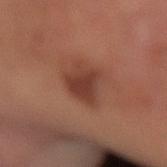follow-up: imaged on a skin check; not biopsied
lesion size: about 4 mm
automated lesion analysis: a footprint of about 9.5 mm², a shape eccentricity near 0.55, and two-axis asymmetry of about 0.25; a lesion color around L≈37 a*≈23 b*≈27 in CIELAB
subject: male, about 65 years old
image source: total-body-photography crop, ~15 mm field of view
location: the left arm
illumination: cross-polarized illumination